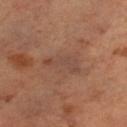Captured during whole-body skin photography for melanoma surveillance; the lesion was not biopsied. A female subject aged approximately 60. This image is a 15 mm lesion crop taken from a total-body photograph. The lesion is located on the left lower leg.